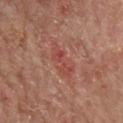<lesion>
  <biopsy_status>not biopsied; imaged during a skin examination</biopsy_status>
  <image>
    <source>total-body photography crop</source>
    <field_of_view_mm>15</field_of_view_mm>
  </image>
  <site>mid back</site>
  <lighting>cross-polarized</lighting>
  <automated_metrics>
    <area_mm2_approx>5.0</area_mm2_approx>
    <shape_asymmetry>0.35</shape_asymmetry>
  </automated_metrics>
  <patient>
    <sex>male</sex>
    <age_approx>65</age_approx>
  </patient>
</lesion>